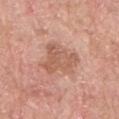Impression:
Part of a total-body skin-imaging series; this lesion was reviewed on a skin check and was not flagged for biopsy.
Background:
A lesion tile, about 15 mm wide, cut from a 3D total-body photograph. Measured at roughly 5 mm in maximum diameter. The total-body-photography lesion software estimated a lesion area of about 13 mm², a shape eccentricity near 0.6, and two-axis asymmetry of about 0.4. The software also gave an average lesion color of about L≈58 a*≈23 b*≈30 (CIELAB), a lesion–skin lightness drop of about 9, and a normalized lesion–skin contrast near 6. It also reported border irregularity of about 4.5 on a 0–10 scale, a within-lesion color-variation index near 3/10, and radial color variation of about 1. The lesion is on the left upper arm. Captured under white-light illumination. A male patient, aged 63 to 67.